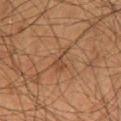Captured during whole-body skin photography for melanoma surveillance; the lesion was not biopsied. The patient is a male aged around 60. The tile uses cross-polarized illumination. A 15 mm close-up tile from a total-body photography series done for melanoma screening. Measured at roughly 3 mm in maximum diameter.